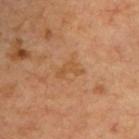This lesion was catalogued during total-body skin photography and was not selected for biopsy. A lesion tile, about 15 mm wide, cut from a 3D total-body photograph. A subject aged around 65. Captured under cross-polarized illumination. Automated image analysis of the tile measured an average lesion color of about L≈52 a*≈22 b*≈39 (CIELAB), a lesion–skin lightness drop of about 6, and a normalized border contrast of about 5.5. From the right upper arm.